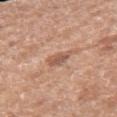biopsy_status: not biopsied; imaged during a skin examination
site: right forearm
image:
  source: total-body photography crop
  field_of_view_mm: 15
patient:
  sex: female
  age_approx: 75
lighting: white-light